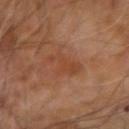Q: Was a biopsy performed?
A: imaged on a skin check; not biopsied
Q: What is the anatomic site?
A: the right arm
Q: Lesion size?
A: ≈4.5 mm
Q: What is the imaging modality?
A: 15 mm crop, total-body photography
Q: Illumination type?
A: cross-polarized illumination
Q: Who is the patient?
A: male, aged 58 to 62
Q: What did automated image analysis measure?
A: border irregularity of about 7 on a 0–10 scale and internal color variation of about 1.5 on a 0–10 scale